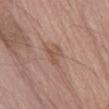follow-up — no biopsy performed (imaged during a skin exam)
TBP lesion metrics — a lesion color around L≈52 a*≈20 b*≈28 in CIELAB, about 7 CIELAB-L* units darker than the surrounding skin, and a normalized border contrast of about 5.5; a border-irregularity rating of about 7/10, a within-lesion color-variation index near 0.5/10, and a peripheral color-asymmetry measure near 0; a classifier nevus-likeness of about 0/100 and a lesion-detection confidence of about 100/100
subject — male, about 70 years old
lighting — white-light
anatomic site — the abdomen
image — ~15 mm tile from a whole-body skin photo